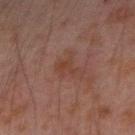Part of a total-body skin-imaging series; this lesion was reviewed on a skin check and was not flagged for biopsy. A lesion tile, about 15 mm wide, cut from a 3D total-body photograph. The lesion is located on the arm. The total-body-photography lesion software estimated a footprint of about 5 mm², an outline eccentricity of about 0.7 (0 = round, 1 = elongated), and a symmetry-axis asymmetry near 0.5. It also reported border irregularity of about 6 on a 0–10 scale, a color-variation rating of about 2/10, and a peripheral color-asymmetry measure near 0.5. The software also gave an automated nevus-likeness rating near 0 out of 100 and a lesion-detection confidence of about 100/100. A male patient aged approximately 30. Measured at roughly 3 mm in maximum diameter.